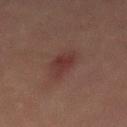Impression: The lesion was tiled from a total-body skin photograph and was not biopsied. Acquisition and patient details: The lesion is located on the mid back. A male patient, aged 43–47. A 15 mm close-up extracted from a 3D total-body photography capture.